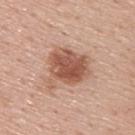Part of a total-body skin-imaging series; this lesion was reviewed on a skin check and was not flagged for biopsy. On the upper back. A lesion tile, about 15 mm wide, cut from a 3D total-body photograph. Automated tile analysis of the lesion measured a lesion area of about 17 mm², an eccentricity of roughly 0.55, and two-axis asymmetry of about 0.35. It also reported a mean CIELAB color near L≈55 a*≈22 b*≈30, about 13 CIELAB-L* units darker than the surrounding skin, and a lesion-to-skin contrast of about 8.5 (normalized; higher = more distinct). It also reported internal color variation of about 5 on a 0–10 scale and radial color variation of about 1.5. The patient is a male approximately 55 years of age. Longest diameter approximately 5.5 mm. Captured under white-light illumination.